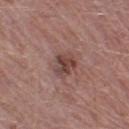Context:
A male subject, aged 48–52. The tile uses white-light illumination. Automated image analysis of the tile measured an area of roughly 6 mm² and a shape eccentricity near 0.55. The analysis additionally found a mean CIELAB color near L≈43 a*≈20 b*≈22, about 10 CIELAB-L* units darker than the surrounding skin, and a normalized lesion–skin contrast near 8. The analysis additionally found a border-irregularity index near 3/10, internal color variation of about 5.5 on a 0–10 scale, and a peripheral color-asymmetry measure near 2. Located on the left thigh. Approximately 3 mm at its widest. Cropped from a whole-body photographic skin survey; the tile spans about 15 mm.